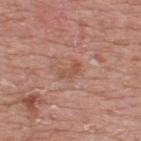Part of a total-body skin-imaging series; this lesion was reviewed on a skin check and was not flagged for biopsy.
On the back.
Imaged with white-light lighting.
A roughly 15 mm field-of-view crop from a total-body skin photograph.
A male subject, roughly 75 years of age.